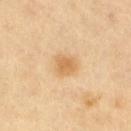This lesion was catalogued during total-body skin photography and was not selected for biopsy. On the right thigh. A female subject, approximately 70 years of age. A roughly 15 mm field-of-view crop from a total-body skin photograph. This is a cross-polarized tile. About 2.5 mm across. The lesion-visualizer software estimated a lesion color around L≈68 a*≈19 b*≈43 in CIELAB, roughly 10 lightness units darker than nearby skin, and a lesion-to-skin contrast of about 6.5 (normalized; higher = more distinct). The analysis additionally found border irregularity of about 2 on a 0–10 scale, internal color variation of about 2.5 on a 0–10 scale, and a peripheral color-asymmetry measure near 1.